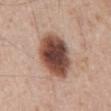Q: Was a biopsy performed?
A: imaged on a skin check; not biopsied